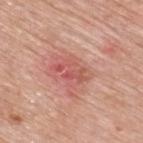notes — total-body-photography surveillance lesion; no biopsy | lighting — white-light illumination | subject — male, aged around 80 | site — the upper back | image — total-body-photography crop, ~15 mm field of view.